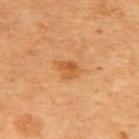Part of a total-body skin-imaging series; this lesion was reviewed on a skin check and was not flagged for biopsy. The lesion is on the upper back. The tile uses cross-polarized illumination. A lesion tile, about 15 mm wide, cut from a 3D total-body photograph. An algorithmic analysis of the crop reported a mean CIELAB color near L≈47 a*≈23 b*≈38, about 8 CIELAB-L* units darker than the surrounding skin, and a lesion-to-skin contrast of about 6.5 (normalized; higher = more distinct). And it measured a border-irregularity rating of about 3.5/10, internal color variation of about 3 on a 0–10 scale, and radial color variation of about 1. Approximately 2.5 mm at its widest. A female subject, in their mid- to late 50s.